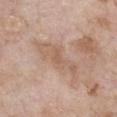Image and clinical context: A 15 mm crop from a total-body photograph taken for skin-cancer surveillance. On the front of the torso. A female patient, in their mid- to late 70s. About 6.5 mm across.